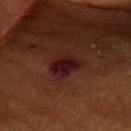Clinical impression:
The lesion was photographed on a routine skin check and not biopsied; there is no pathology result.
Background:
On the head or neck. A female subject, aged approximately 80. Measured at roughly 3.5 mm in maximum diameter. A close-up tile cropped from a whole-body skin photograph, about 15 mm across.